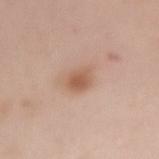| field | value |
|---|---|
| biopsy status | total-body-photography surveillance lesion; no biopsy |
| tile lighting | white-light illumination |
| image-analysis metrics | an average lesion color of about L≈58 a*≈20 b*≈30 (CIELAB), roughly 10 lightness units darker than nearby skin, and a lesion-to-skin contrast of about 7 (normalized; higher = more distinct); border irregularity of about 2 on a 0–10 scale and a peripheral color-asymmetry measure near 1; a nevus-likeness score of about 90/100 |
| site | the right forearm |
| image source | ~15 mm crop, total-body skin-cancer survey |
| patient | female, about 65 years old |
| size | ≈3 mm |